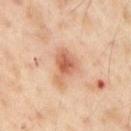The lesion was tiled from a total-body skin photograph and was not biopsied. A male patient aged 53–57. The lesion is on the right upper arm. The tile uses cross-polarized illumination. Longest diameter approximately 4.5 mm. A roughly 15 mm field-of-view crop from a total-body skin photograph.